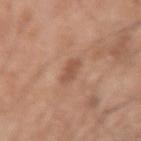This lesion was catalogued during total-body skin photography and was not selected for biopsy. On the right upper arm. The lesion-visualizer software estimated an area of roughly 4 mm² and an eccentricity of roughly 0.75. And it measured a lesion color around L≈53 a*≈22 b*≈30 in CIELAB, about 9 CIELAB-L* units darker than the surrounding skin, and a normalized border contrast of about 6. It also reported a border-irregularity index near 2/10. It also reported an automated nevus-likeness rating near 0 out of 100 and lesion-presence confidence of about 100/100. The recorded lesion diameter is about 2.5 mm. A lesion tile, about 15 mm wide, cut from a 3D total-body photograph. A male subject, about 55 years old.